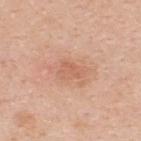Notes:
– follow-up: no biopsy performed (imaged during a skin exam)
– automated lesion analysis: an average lesion color of about L≈62 a*≈23 b*≈33 (CIELAB), about 7 CIELAB-L* units darker than the surrounding skin, and a lesion-to-skin contrast of about 5 (normalized; higher = more distinct); a border-irregularity rating of about 3/10, a color-variation rating of about 2/10, and peripheral color asymmetry of about 0.5
– body site: the upper back
– diameter: ~3 mm (longest diameter)
– subject: female, about 30 years old
– acquisition: ~15 mm tile from a whole-body skin photo